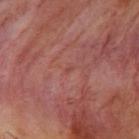This lesion was catalogued during total-body skin photography and was not selected for biopsy. A male patient in their mid-50s. A 15 mm crop from a total-body photograph taken for skin-cancer surveillance. Captured under cross-polarized illumination. The lesion is located on the right upper arm. About 1 mm across. The total-body-photography lesion software estimated a nevus-likeness score of about 0/100 and a detector confidence of about 100 out of 100 that the crop contains a lesion.